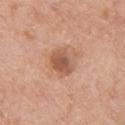Impression: This lesion was catalogued during total-body skin photography and was not selected for biopsy. Acquisition and patient details: The recorded lesion diameter is about 3.5 mm. A 15 mm crop from a total-body photograph taken for skin-cancer surveillance. The lesion is located on the upper back. Imaged with white-light lighting. A male patient roughly 60 years of age.